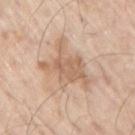follow-up: no biopsy performed (imaged during a skin exam)
patient: male, about 70 years old
acquisition: ~15 mm tile from a whole-body skin photo
location: the right upper arm
size: ~6.5 mm (longest diameter)
lighting: white-light